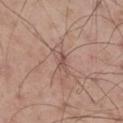Notes:
– biopsy status — imaged on a skin check; not biopsied
– image — total-body-photography crop, ~15 mm field of view
– site — the leg
– lesion diameter — ~2.5 mm (longest diameter)
– illumination — white-light illumination
– patient — male, in their mid-50s